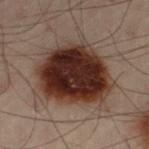Impression: This lesion was catalogued during total-body skin photography and was not selected for biopsy. Background: On the left thigh. The recorded lesion diameter is about 8 mm. A male patient aged approximately 50. Cropped from a total-body skin-imaging series; the visible field is about 15 mm. Imaged with cross-polarized lighting.